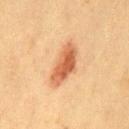{"biopsy_status": "not biopsied; imaged during a skin examination", "site": "leg", "lesion_size": {"long_diameter_mm_approx": 5.5}, "patient": {"sex": "female", "age_approx": 40}, "image": {"source": "total-body photography crop", "field_of_view_mm": 15}, "automated_metrics": {"area_mm2_approx": 11.0, "shape_asymmetry": 0.3, "border_irregularity_0_10": 3.0, "color_variation_0_10": 5.0, "peripheral_color_asymmetry": 1.5, "nevus_likeness_0_100": 95}}